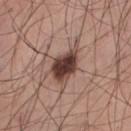No biopsy was performed on this lesion — it was imaged during a full skin examination and was not determined to be concerning.
A lesion tile, about 15 mm wide, cut from a 3D total-body photograph.
The lesion is located on the chest.
The tile uses white-light illumination.
A male subject aged 28 to 32.
Measured at roughly 5.5 mm in maximum diameter.